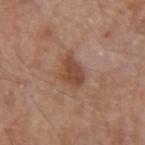Clinical impression:
Captured during whole-body skin photography for melanoma surveillance; the lesion was not biopsied.
Clinical summary:
Captured under white-light illumination. Cropped from a total-body skin-imaging series; the visible field is about 15 mm. The subject is a male roughly 80 years of age. An algorithmic analysis of the crop reported an eccentricity of roughly 0.75 and two-axis asymmetry of about 0.3. The software also gave a border-irregularity rating of about 3/10, a within-lesion color-variation index near 2.5/10, and radial color variation of about 1. Longest diameter approximately 4 mm. Located on the left upper arm.